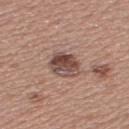Impression: Captured during whole-body skin photography for melanoma surveillance; the lesion was not biopsied. Background: This image is a 15 mm lesion crop taken from a total-body photograph. Captured under white-light illumination. The lesion is on the upper back. A female subject approximately 50 years of age.